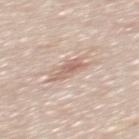| field | value |
|---|---|
| workup | no biopsy performed (imaged during a skin exam) |